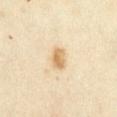{"lighting": "cross-polarized", "image": {"source": "total-body photography crop", "field_of_view_mm": 15}, "lesion_size": {"long_diameter_mm_approx": 2.5}, "site": "abdomen", "patient": {"sex": "female", "age_approx": 35}}